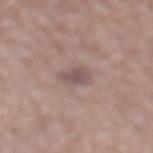A region of skin cropped from a whole-body photographic capture, roughly 15 mm wide. Captured under white-light illumination. A female patient, in their mid- to late 60s. From the back. Automated tile analysis of the lesion measured a mean CIELAB color near L≈51 a*≈15 b*≈18, about 10 CIELAB-L* units darker than the surrounding skin, and a lesion-to-skin contrast of about 7 (normalized; higher = more distinct). The analysis additionally found a lesion-detection confidence of about 100/100.